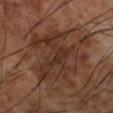• size — ~7.5 mm (longest diameter)
• patient — male, about 70 years old
• image-analysis metrics — about 7 CIELAB-L* units darker than the surrounding skin and a lesion-to-skin contrast of about 6.5 (normalized; higher = more distinct); a border-irregularity rating of about 9/10, internal color variation of about 5 on a 0–10 scale, and peripheral color asymmetry of about 1.5
• body site — the arm
• illumination — cross-polarized illumination
• image source — total-body-photography crop, ~15 mm field of view